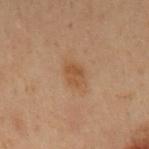biopsy status=no biopsy performed (imaged during a skin exam)
patient=male, aged around 55
body site=the right upper arm
lesion diameter=~2.5 mm (longest diameter)
acquisition=~15 mm crop, total-body skin-cancer survey